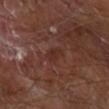workup — imaged on a skin check; not biopsied
subject — male, aged approximately 65
location — the arm
TBP lesion metrics — an eccentricity of roughly 0.8; a nevus-likeness score of about 15/100 and a lesion-detection confidence of about 100/100
imaging modality — ~15 mm crop, total-body skin-cancer survey
size — ≈3 mm